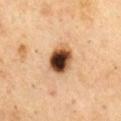Imaged with cross-polarized lighting. Measured at roughly 4 mm in maximum diameter. A male patient, about 70 years old. On the chest. This image is a 15 mm lesion crop taken from a total-body photograph.